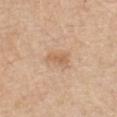biopsy status = total-body-photography surveillance lesion; no biopsy
automated lesion analysis = a border-irregularity index near 3.5/10, a color-variation rating of about 1/10, and peripheral color asymmetry of about 0.5; a classifier nevus-likeness of about 5/100
subject = male, about 65 years old
image = 15 mm crop, total-body photography
body site = the front of the torso
lighting = white-light illumination